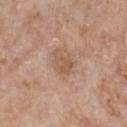Assessment:
No biopsy was performed on this lesion — it was imaged during a full skin examination and was not determined to be concerning.
Background:
On the chest. Approximately 3 mm at its widest. A close-up tile cropped from a whole-body skin photograph, about 15 mm across. The patient is a female aged 58–62. Automated tile analysis of the lesion measured a lesion area of about 4 mm² and a symmetry-axis asymmetry near 0.45.